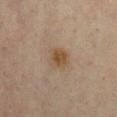{"biopsy_status": "not biopsied; imaged during a skin examination", "lesion_size": {"long_diameter_mm_approx": 3.0}, "patient": {"sex": "male", "age_approx": 45}, "site": "front of the torso", "image": {"source": "total-body photography crop", "field_of_view_mm": 15}, "lighting": "cross-polarized"}